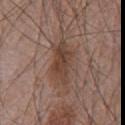Captured during whole-body skin photography for melanoma surveillance; the lesion was not biopsied.
A region of skin cropped from a whole-body photographic capture, roughly 15 mm wide.
A male patient about 70 years old.
The lesion's longest dimension is about 5.5 mm.
Captured under white-light illumination.
From the chest.